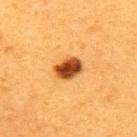Assessment:
The lesion was photographed on a routine skin check and not biopsied; there is no pathology result.
Clinical summary:
A roughly 15 mm field-of-view crop from a total-body skin photograph. A female patient, roughly 40 years of age. Automated tile analysis of the lesion measured an area of roughly 7 mm², an outline eccentricity of about 0.7 (0 = round, 1 = elongated), and two-axis asymmetry of about 0.15. The software also gave a lesion color around L≈41 a*≈25 b*≈40 in CIELAB, about 19 CIELAB-L* units darker than the surrounding skin, and a lesion-to-skin contrast of about 14 (normalized; higher = more distinct). The tile uses cross-polarized illumination. On the upper back. Measured at roughly 3.5 mm in maximum diameter.